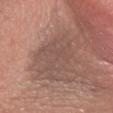workup = total-body-photography surveillance lesion; no biopsy
acquisition = 15 mm crop, total-body photography
location = the right forearm
subject = female, about 50 years old
lighting = white-light illumination